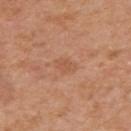Assessment:
The lesion was photographed on a routine skin check and not biopsied; there is no pathology result.
Image and clinical context:
Cropped from a whole-body photographic skin survey; the tile spans about 15 mm. Measured at roughly 2.5 mm in maximum diameter. The tile uses white-light illumination. A male subject in their mid- to late 70s. The lesion is on the right upper arm.